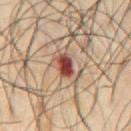Assessment: Captured during whole-body skin photography for melanoma surveillance; the lesion was not biopsied. Context: The lesion is on the abdomen. The total-body-photography lesion software estimated an eccentricity of roughly 0.7. A roughly 15 mm field-of-view crop from a total-body skin photograph. A male subject aged around 50. This is a cross-polarized tile.